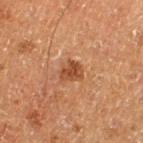<record>
<biopsy_status>not biopsied; imaged during a skin examination</biopsy_status>
<lighting>cross-polarized</lighting>
<patient>
  <sex>male</sex>
  <age_approx>75</age_approx>
</patient>
<site>left thigh</site>
<image>
  <source>total-body photography crop</source>
  <field_of_view_mm>15</field_of_view_mm>
</image>
</record>